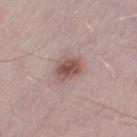The tile uses white-light illumination. On the left thigh. A 15 mm close-up tile from a total-body photography series done for melanoma screening. About 3 mm across. The subject is a male roughly 60 years of age. An algorithmic analysis of the crop reported a detector confidence of about 100 out of 100 that the crop contains a lesion.